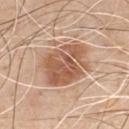Q: Was this lesion biopsied?
A: imaged on a skin check; not biopsied
Q: Automated lesion metrics?
A: an area of roughly 23 mm², an outline eccentricity of about 0.6 (0 = round, 1 = elongated), and a symmetry-axis asymmetry near 0.2; border irregularity of about 3 on a 0–10 scale, internal color variation of about 5 on a 0–10 scale, and a peripheral color-asymmetry measure near 2; an automated nevus-likeness rating near 80 out of 100 and a detector confidence of about 100 out of 100 that the crop contains a lesion
Q: What are the patient's age and sex?
A: male, about 50 years old
Q: How was this image acquired?
A: ~15 mm crop, total-body skin-cancer survey
Q: Lesion location?
A: the chest
Q: Illumination type?
A: white-light